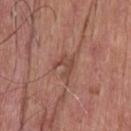Impression: No biopsy was performed on this lesion — it was imaged during a full skin examination and was not determined to be concerning. Clinical summary: Cropped from a total-body skin-imaging series; the visible field is about 15 mm. The lesion is on the head or neck. An algorithmic analysis of the crop reported a detector confidence of about 65 out of 100 that the crop contains a lesion. The patient is a male aged 58 to 62. Captured under white-light illumination.